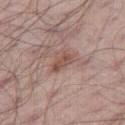Captured during whole-body skin photography for melanoma surveillance; the lesion was not biopsied. Automated image analysis of the tile measured a border-irregularity rating of about 3.5/10, a within-lesion color-variation index near 2.5/10, and radial color variation of about 1. The subject is a male about 55 years old. A 15 mm close-up tile from a total-body photography series done for melanoma screening. About 3 mm across. Located on the right thigh. Captured under white-light illumination.